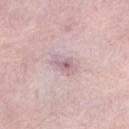An algorithmic analysis of the crop reported a footprint of about 5 mm². The software also gave an average lesion color of about L≈65 a*≈19 b*≈16 (CIELAB), roughly 9 lightness units darker than nearby skin, and a lesion-to-skin contrast of about 6 (normalized; higher = more distinct). And it measured border irregularity of about 2.5 on a 0–10 scale and a within-lesion color-variation index near 6.5/10. A 15 mm crop from a total-body photograph taken for skin-cancer surveillance. A female subject, about 60 years old. Approximately 3.5 mm at its widest. The lesion is located on the abdomen.